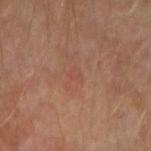workup = no biopsy performed (imaged during a skin exam)
automated metrics = a within-lesion color-variation index near 0/10 and a peripheral color-asymmetry measure near 0
body site = the right forearm
lesion diameter = about 1.5 mm
patient = in their mid- to late 50s
image source = 15 mm crop, total-body photography